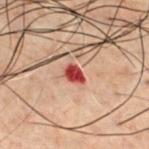Case summary:
- biopsy status · total-body-photography surveillance lesion; no biopsy
- anatomic site · the chest
- patient · male, aged approximately 60
- image source · ~15 mm tile from a whole-body skin photo